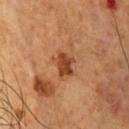workup: catalogued during a skin exam; not biopsied
body site: the chest
subject: male, aged approximately 55
automated lesion analysis: a shape-asymmetry score of about 0.3 (0 = symmetric); a border-irregularity index near 3/10, internal color variation of about 3 on a 0–10 scale, and a peripheral color-asymmetry measure near 1
acquisition: total-body-photography crop, ~15 mm field of view
tile lighting: cross-polarized